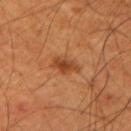Recorded during total-body skin imaging; not selected for excision or biopsy.
Cropped from a whole-body photographic skin survey; the tile spans about 15 mm.
This is a cross-polarized tile.
Measured at roughly 3 mm in maximum diameter.
Located on the right upper arm.
The subject is a male about 70 years old.
The lesion-visualizer software estimated an area of roughly 5 mm², an eccentricity of roughly 0.7, and two-axis asymmetry of about 0.25. The software also gave a lesion color around L≈43 a*≈25 b*≈38 in CIELAB, a lesion–skin lightness drop of about 9, and a lesion-to-skin contrast of about 7.5 (normalized; higher = more distinct). The software also gave a border-irregularity index near 2.5/10, a within-lesion color-variation index near 3.5/10, and a peripheral color-asymmetry measure near 1.